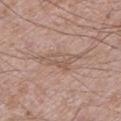The lesion was tiled from a total-body skin photograph and was not biopsied. A close-up tile cropped from a whole-body skin photograph, about 15 mm across. On the left lower leg. About 5.5 mm across. The patient is a male approximately 60 years of age.